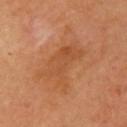Part of a total-body skin-imaging series; this lesion was reviewed on a skin check and was not flagged for biopsy.
Located on the left upper arm.
Cropped from a total-body skin-imaging series; the visible field is about 15 mm.
A female subject, aged around 60.
This is a cross-polarized tile.
The recorded lesion diameter is about 6 mm.